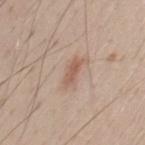<case>
<biopsy_status>not biopsied; imaged during a skin examination</biopsy_status>
<image>
  <source>total-body photography crop</source>
  <field_of_view_mm>15</field_of_view_mm>
</image>
<site>chest</site>
<lighting>white-light</lighting>
<patient>
  <sex>male</sex>
  <age_approx>45</age_approx>
</patient>
<lesion_size>
  <long_diameter_mm_approx>3.0</long_diameter_mm_approx>
</lesion_size>
</case>